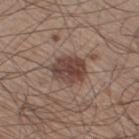The lesion-visualizer software estimated a mean CIELAB color near L≈42 a*≈17 b*≈22, roughly 12 lightness units darker than nearby skin, and a normalized lesion–skin contrast near 9.5. The analysis additionally found a nevus-likeness score of about 80/100 and lesion-presence confidence of about 100/100. A male subject aged 58–62. Longest diameter approximately 4.5 mm. From the right thigh. A close-up tile cropped from a whole-body skin photograph, about 15 mm across.